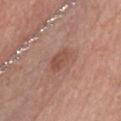Clinical impression: This lesion was catalogued during total-body skin photography and was not selected for biopsy. Context: A female subject aged 58 to 62. An algorithmic analysis of the crop reported a footprint of about 5 mm², a shape eccentricity near 0.85, and a shape-asymmetry score of about 0.25 (0 = symmetric). The analysis additionally found a normalized border contrast of about 6. It also reported border irregularity of about 2.5 on a 0–10 scale. The software also gave a nevus-likeness score of about 20/100. On the chest. This is a white-light tile. A roughly 15 mm field-of-view crop from a total-body skin photograph. The lesion's longest dimension is about 3 mm.